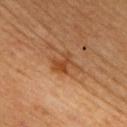Q: Was this lesion biopsied?
A: no biopsy performed (imaged during a skin exam)
Q: Illumination type?
A: cross-polarized
Q: What did automated image analysis measure?
A: an average lesion color of about L≈40 a*≈22 b*≈34 (CIELAB), a lesion–skin lightness drop of about 8, and a lesion-to-skin contrast of about 7 (normalized; higher = more distinct)
Q: Who is the patient?
A: male, aged 53 to 57
Q: Lesion location?
A: the chest
Q: Lesion size?
A: ≈3 mm
Q: How was this image acquired?
A: total-body-photography crop, ~15 mm field of view